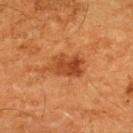biopsy_status: not biopsied; imaged during a skin examination
site: upper back
patient:
  sex: male
  age_approx: 65
automated_metrics:
  area_mm2_approx: 9.5
  eccentricity: 0.85
  cielab_L: 38
  cielab_a: 25
  cielab_b: 35
  vs_skin_darker_L: 9.0
  vs_skin_contrast_norm: 8.0
  border_irregularity_0_10: 4.5
  color_variation_0_10: 3.5
  peripheral_color_asymmetry: 1.5
  nevus_likeness_0_100: 80
  lesion_detection_confidence_0_100: 100
image:
  source: total-body photography crop
  field_of_view_mm: 15